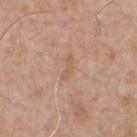Recorded during total-body skin imaging; not selected for excision or biopsy. Approximately 3 mm at its widest. This is a white-light tile. A male patient, aged 48–52. The total-body-photography lesion software estimated a lesion area of about 3 mm² and an outline eccentricity of about 0.95 (0 = round, 1 = elongated). The software also gave a lesion color around L≈59 a*≈19 b*≈32 in CIELAB, roughly 6 lightness units darker than nearby skin, and a normalized lesion–skin contrast near 5. The analysis additionally found a border-irregularity rating of about 4/10, internal color variation of about 0 on a 0–10 scale, and radial color variation of about 0. The software also gave a classifier nevus-likeness of about 0/100 and lesion-presence confidence of about 100/100. The lesion is located on the chest. A roughly 15 mm field-of-view crop from a total-body skin photograph.